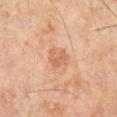<case>
  <biopsy_status>not biopsied; imaged during a skin examination</biopsy_status>
  <patient>
    <sex>male</sex>
    <age_approx>45</age_approx>
  </patient>
  <lighting>cross-polarized</lighting>
  <image>
    <source>total-body photography crop</source>
    <field_of_view_mm>15</field_of_view_mm>
  </image>
  <automated_metrics>
    <border_irregularity_0_10>3.0</border_irregularity_0_10>
    <peripheral_color_asymmetry>0.5</peripheral_color_asymmetry>
    <nevus_likeness_0_100>0</nevus_likeness_0_100>
    <lesion_detection_confidence_0_100>100</lesion_detection_confidence_0_100>
  </automated_metrics>
  <lesion_size>
    <long_diameter_mm_approx>2.5</long_diameter_mm_approx>
  </lesion_size>
  <site>right lower leg</site>
</case>